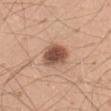A region of skin cropped from a whole-body photographic capture, roughly 15 mm wide. The recorded lesion diameter is about 4 mm. The lesion is on the front of the torso. A male subject approximately 50 years of age. Captured under white-light illumination.